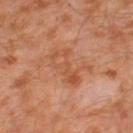| field | value |
|---|---|
| notes | catalogued during a skin exam; not biopsied |
| patient | male, aged 28 to 32 |
| anatomic site | the left thigh |
| diameter | ~5 mm (longest diameter) |
| image source | 15 mm crop, total-body photography |
| illumination | cross-polarized illumination |